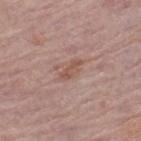workup — no biopsy performed (imaged during a skin exam) | lesion size — ~3 mm (longest diameter) | TBP lesion metrics — a lesion color around L≈53 a*≈21 b*≈26 in CIELAB and about 8 CIELAB-L* units darker than the surrounding skin; a border-irregularity index near 4/10, a color-variation rating of about 0/10, and peripheral color asymmetry of about 0; an automated nevus-likeness rating near 0 out of 100 | imaging modality — ~15 mm tile from a whole-body skin photo | body site — the left thigh | subject — male, about 75 years old | illumination — white-light.